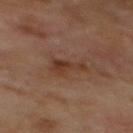follow-up: catalogued during a skin exam; not biopsied
subject: male, in their 70s
illumination: cross-polarized
site: the back
imaging modality: total-body-photography crop, ~15 mm field of view
size: ≈3.5 mm
TBP lesion metrics: an automated nevus-likeness rating near 15 out of 100 and lesion-presence confidence of about 100/100